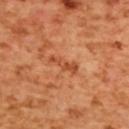* biopsy status: imaged on a skin check; not biopsied
* subject: female, roughly 55 years of age
* image source: ~15 mm crop, total-body skin-cancer survey
* lighting: cross-polarized illumination
* body site: the upper back
* automated metrics: a footprint of about 4 mm², an outline eccentricity of about 0.95 (0 = round, 1 = elongated), and two-axis asymmetry of about 0.35; an average lesion color of about L≈52 a*≈31 b*≈42 (CIELAB), a lesion–skin lightness drop of about 9, and a lesion-to-skin contrast of about 6.5 (normalized; higher = more distinct); a border-irregularity rating of about 4.5/10 and peripheral color asymmetry of about 0; a nevus-likeness score of about 0/100 and lesion-presence confidence of about 100/100